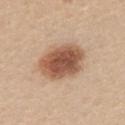This image is a 15 mm lesion crop taken from a total-body photograph.
A female subject aged around 25.
From the upper back.
The tile uses white-light illumination.
The total-body-photography lesion software estimated a lesion color around L≈54 a*≈21 b*≈31 in CIELAB, a lesion–skin lightness drop of about 16, and a normalized border contrast of about 10.5. The software also gave a classifier nevus-likeness of about 100/100 and lesion-presence confidence of about 100/100.
About 5.5 mm across.